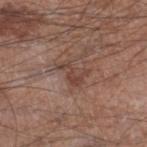biopsy status = total-body-photography surveillance lesion; no biopsy | lesion diameter = ≈3.5 mm | lighting = white-light | site = the right lower leg | patient = male, aged 53–57 | acquisition = ~15 mm crop, total-body skin-cancer survey.